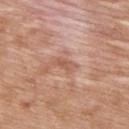Assessment: Part of a total-body skin-imaging series; this lesion was reviewed on a skin check and was not flagged for biopsy. Acquisition and patient details: Cropped from a total-body skin-imaging series; the visible field is about 15 mm. The total-body-photography lesion software estimated a lesion area of about 3 mm², an eccentricity of roughly 0.85, and a shape-asymmetry score of about 0.35 (0 = symmetric). A male subject, aged approximately 50. Approximately 2.5 mm at its widest. The tile uses white-light illumination. Located on the upper back.